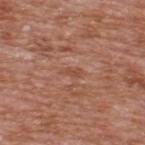biopsy status — no biopsy performed (imaged during a skin exam)
lighting — white-light illumination
location — the upper back
image-analysis metrics — border irregularity of about 3 on a 0–10 scale, a within-lesion color-variation index near 0/10, and radial color variation of about 0
lesion size — ~2.5 mm (longest diameter)
acquisition — ~15 mm tile from a whole-body skin photo
patient — male, in their mid-60s